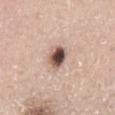Case summary:
- workup · total-body-photography surveillance lesion; no biopsy
- body site · the mid back
- patient · male, aged 38 to 42
- imaging modality · 15 mm crop, total-body photography
- lesion diameter · ≈3 mm
- illumination · white-light